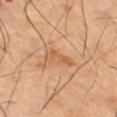  biopsy_status: not biopsied; imaged during a skin examination
  lesion_size:
    long_diameter_mm_approx: 3.5
  lighting: cross-polarized
  site: right thigh
  automated_metrics:
    border_irregularity_0_10: 4.0
    color_variation_0_10: 3.0
    peripheral_color_asymmetry: 1.0
    nevus_likeness_0_100: 0
    lesion_detection_confidence_0_100: 100
  image:
    source: total-body photography crop
    field_of_view_mm: 15
  patient:
    sex: male
    age_approx: 65